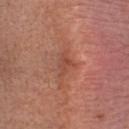| key | value |
|---|---|
| notes | imaged on a skin check; not biopsied |
| subject | female, aged approximately 30 |
| illumination | white-light illumination |
| automated metrics | an area of roughly 3.5 mm², a shape eccentricity near 0.75, and two-axis asymmetry of about 0.4; an automated nevus-likeness rating near 0 out of 100 |
| acquisition | ~15 mm crop, total-body skin-cancer survey |
| body site | the head or neck |
| lesion diameter | ~3 mm (longest diameter) |